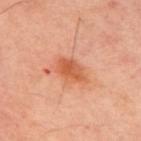follow-up: imaged on a skin check; not biopsied | TBP lesion metrics: a lesion area of about 6.5 mm² and a shape eccentricity near 0.75 | patient: male, in their 50s | tile lighting: cross-polarized | lesion size: about 3.5 mm | image source: total-body-photography crop, ~15 mm field of view | anatomic site: the upper back.